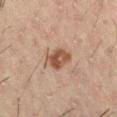– workup — imaged on a skin check; not biopsied
– lesion diameter — ≈3.5 mm
– patient — male, in their 50s
– image source — total-body-photography crop, ~15 mm field of view
– location — the right thigh
– lighting — cross-polarized